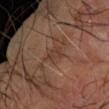A male subject aged approximately 65. From the right forearm. Cropped from a whole-body photographic skin survey; the tile spans about 15 mm. Approximately 3 mm at its widest.